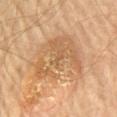Part of a total-body skin-imaging series; this lesion was reviewed on a skin check and was not flagged for biopsy. The lesion's longest dimension is about 7.5 mm. A male subject, approximately 65 years of age. Located on the chest. The tile uses cross-polarized illumination. This image is a 15 mm lesion crop taken from a total-body photograph.